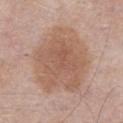biopsy status=total-body-photography surveillance lesion; no biopsy
site=the abdomen
imaging modality=~15 mm tile from a whole-body skin photo
subject=male, aged around 55